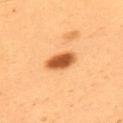This lesion was catalogued during total-body skin photography and was not selected for biopsy. A male patient, aged 53–57. The total-body-photography lesion software estimated a footprint of about 7 mm² and a shape-asymmetry score of about 0.15 (0 = symmetric). A lesion tile, about 15 mm wide, cut from a 3D total-body photograph. The lesion is located on the upper back. The tile uses cross-polarized illumination. About 3.5 mm across.